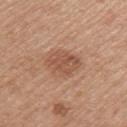No biopsy was performed on this lesion — it was imaged during a full skin examination and was not determined to be concerning.
A female patient, in their 50s.
Located on the left upper arm.
A lesion tile, about 15 mm wide, cut from a 3D total-body photograph.